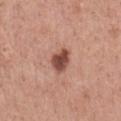Notes:
- biopsy status · imaged on a skin check; not biopsied
- anatomic site · the front of the torso
- lighting · white-light illumination
- patient · male, in their mid- to late 40s
- acquisition · total-body-photography crop, ~15 mm field of view